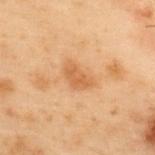follow-up: total-body-photography surveillance lesion; no biopsy
lesion size: ~3.5 mm (longest diameter)
patient: male, in their mid- to late 50s
image: 15 mm crop, total-body photography
illumination: cross-polarized
automated lesion analysis: border irregularity of about 3.5 on a 0–10 scale and a within-lesion color-variation index near 1.5/10; a nevus-likeness score of about 0/100 and a lesion-detection confidence of about 100/100
body site: the upper back